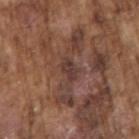Assessment:
This lesion was catalogued during total-body skin photography and was not selected for biopsy.
Context:
About 2.5 mm across. A male patient, in their mid- to late 70s. The tile uses white-light illumination. The total-body-photography lesion software estimated border irregularity of about 3 on a 0–10 scale and radial color variation of about 0.5. Cropped from a whole-body photographic skin survey; the tile spans about 15 mm. From the right upper arm.